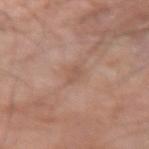{
  "biopsy_status": "not biopsied; imaged during a skin examination",
  "image": {
    "source": "total-body photography crop",
    "field_of_view_mm": 15
  },
  "automated_metrics": {
    "cielab_L": 54,
    "cielab_a": 19,
    "cielab_b": 27,
    "vs_skin_darker_L": 7.0,
    "vs_skin_contrast_norm": 5.0,
    "color_variation_0_10": 0.0
  },
  "lesion_size": {
    "long_diameter_mm_approx": 2.5
  },
  "lighting": "white-light",
  "site": "right forearm",
  "patient": {
    "sex": "male",
    "age_approx": 80
  }
}